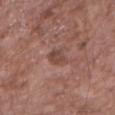The lesion was tiled from a total-body skin photograph and was not biopsied.
Captured under white-light illumination.
The lesion's longest dimension is about 3 mm.
From the right upper arm.
The total-body-photography lesion software estimated about 8 CIELAB-L* units darker than the surrounding skin and a lesion-to-skin contrast of about 6 (normalized; higher = more distinct). The software also gave a within-lesion color-variation index near 2/10 and peripheral color asymmetry of about 0.5. It also reported an automated nevus-likeness rating near 0 out of 100 and a lesion-detection confidence of about 100/100.
A female subject, aged 68 to 72.
A 15 mm close-up tile from a total-body photography series done for melanoma screening.